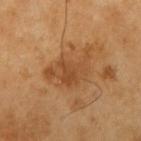Q: Was this lesion biopsied?
A: imaged on a skin check; not biopsied